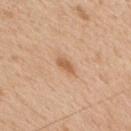Image and clinical context: The tile uses white-light illumination. The lesion is on the upper back. A close-up tile cropped from a whole-body skin photograph, about 15 mm across. Longest diameter approximately 2.5 mm. A male subject in their mid-60s.